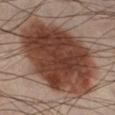Recorded during total-body skin imaging; not selected for excision or biopsy. Located on the right lower leg. A male patient, about 40 years old. The lesion-visualizer software estimated a mean CIELAB color near L≈37 a*≈21 b*≈26. The analysis additionally found peripheral color asymmetry of about 2. A region of skin cropped from a whole-body photographic capture, roughly 15 mm wide.